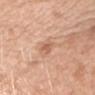Assessment:
Part of a total-body skin-imaging series; this lesion was reviewed on a skin check and was not flagged for biopsy.
Acquisition and patient details:
A lesion tile, about 15 mm wide, cut from a 3D total-body photograph. The tile uses white-light illumination. About 3 mm across. The lesion is on the right upper arm. The patient is a female in their 50s.